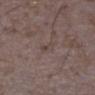{
  "biopsy_status": "not biopsied; imaged during a skin examination",
  "automated_metrics": {
    "cielab_L": 42,
    "cielab_a": 14,
    "cielab_b": 19,
    "vs_skin_darker_L": 5.0,
    "border_irregularity_0_10": 4.0,
    "peripheral_color_asymmetry": 0.0,
    "nevus_likeness_0_100": 0,
    "lesion_detection_confidence_0_100": 95
  },
  "patient": {
    "sex": "female",
    "age_approx": 35
  },
  "site": "right lower leg",
  "lesion_size": {
    "long_diameter_mm_approx": 2.5
  },
  "lighting": "white-light",
  "image": {
    "source": "total-body photography crop",
    "field_of_view_mm": 15
  }
}